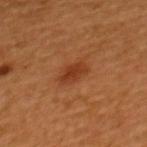This lesion was catalogued during total-body skin photography and was not selected for biopsy.
An algorithmic analysis of the crop reported a border-irregularity index near 2/10, internal color variation of about 1.5 on a 0–10 scale, and peripheral color asymmetry of about 0.5. And it measured an automated nevus-likeness rating near 75 out of 100.
On the back.
The recorded lesion diameter is about 3 mm.
Imaged with cross-polarized lighting.
The subject is a male approximately 45 years of age.
A roughly 15 mm field-of-view crop from a total-body skin photograph.